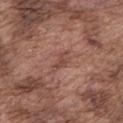Findings:
• notes · imaged on a skin check; not biopsied
• body site · the mid back
• diameter · about 3.5 mm
• illumination · white-light illumination
• image · total-body-photography crop, ~15 mm field of view
• subject · male, about 75 years old